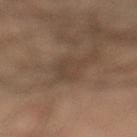The lesion is located on the left lower leg. A male subject, roughly 35 years of age. A 15 mm close-up extracted from a 3D total-body photography capture.